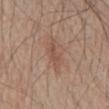<tbp_lesion>
  <biopsy_status>not biopsied; imaged during a skin examination</biopsy_status>
  <image>
    <source>total-body photography crop</source>
    <field_of_view_mm>15</field_of_view_mm>
  </image>
  <patient>
    <sex>male</sex>
    <age_approx>80</age_approx>
  </patient>
  <automated_metrics>
    <eccentricity>0.95</eccentricity>
    <shape_asymmetry>0.35</shape_asymmetry>
    <border_irregularity_0_10>4.5</border_irregularity_0_10>
    <color_variation_0_10>0.5</color_variation_0_10>
    <peripheral_color_asymmetry>0.0</peripheral_color_asymmetry>
    <nevus_likeness_0_100>0</nevus_likeness_0_100>
  </automated_metrics>
  <site>abdomen</site>
  <lighting>white-light</lighting>
</tbp_lesion>